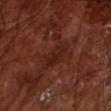This lesion was catalogued during total-body skin photography and was not selected for biopsy.